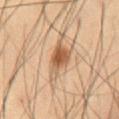biopsy status: catalogued during a skin exam; not biopsied | lesion diameter: ~4 mm (longest diameter) | patient: male, aged around 55 | body site: the front of the torso | tile lighting: cross-polarized | image: total-body-photography crop, ~15 mm field of view.